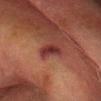Notes:
* notes: total-body-photography surveillance lesion; no biopsy
* size: about 3 mm
* illumination: cross-polarized
* image-analysis metrics: a mean CIELAB color near L≈28 a*≈25 b*≈20, a lesion–skin lightness drop of about 10, and a lesion-to-skin contrast of about 10.5 (normalized; higher = more distinct); a color-variation rating of about 2.5/10 and peripheral color asymmetry of about 0.5; a classifier nevus-likeness of about 15/100 and a lesion-detection confidence of about 100/100
* imaging modality: total-body-photography crop, ~15 mm field of view
* anatomic site: the head or neck
* subject: male, aged approximately 70